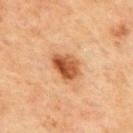The lesion was tiled from a total-body skin photograph and was not biopsied. Located on the mid back. A male subject, aged 68–72. Imaged with cross-polarized lighting. Measured at roughly 4 mm in maximum diameter. A region of skin cropped from a whole-body photographic capture, roughly 15 mm wide. Automated tile analysis of the lesion measured a footprint of about 9 mm² and two-axis asymmetry of about 0.25. The software also gave about 13 CIELAB-L* units darker than the surrounding skin and a normalized border contrast of about 10.